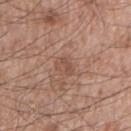This lesion was catalogued during total-body skin photography and was not selected for biopsy.
A 15 mm close-up extracted from a 3D total-body photography capture.
A male patient aged around 65.
The lesion is on the right upper arm.